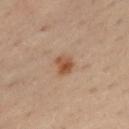Captured during whole-body skin photography for melanoma surveillance; the lesion was not biopsied. A roughly 15 mm field-of-view crop from a total-body skin photograph. The lesion is on the back. The subject is a male approximately 50 years of age. The lesion-visualizer software estimated a lesion area of about 4.5 mm² and an outline eccentricity of about 0.6 (0 = round, 1 = elongated). And it measured a nevus-likeness score of about 90/100 and lesion-presence confidence of about 100/100.